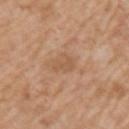Assessment:
Part of a total-body skin-imaging series; this lesion was reviewed on a skin check and was not flagged for biopsy.
Context:
The patient is a male in their 60s. Captured under white-light illumination. Measured at roughly 3 mm in maximum diameter. The lesion is on the arm. A roughly 15 mm field-of-view crop from a total-body skin photograph.